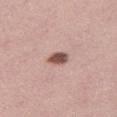workup: catalogued during a skin exam; not biopsied | illumination: white-light | body site: the right thigh | lesion diameter: ≈2.5 mm | subject: female, in their mid- to late 30s | image: 15 mm crop, total-body photography.